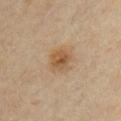Case summary:
* workup: no biopsy performed (imaged during a skin exam)
* site: the chest
* image: total-body-photography crop, ~15 mm field of view
* lighting: cross-polarized
* patient: male, aged 43–47